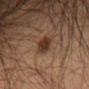Q: Was this lesion biopsied?
A: catalogued during a skin exam; not biopsied
Q: What is the imaging modality?
A: ~15 mm crop, total-body skin-cancer survey
Q: Who is the patient?
A: male, approximately 35 years of age
Q: Where on the body is the lesion?
A: the lower back
Q: What did automated image analysis measure?
A: a lesion area of about 5.5 mm² and an outline eccentricity of about 0.85 (0 = round, 1 = elongated); an average lesion color of about L≈31 a*≈17 b*≈25 (CIELAB), roughly 10 lightness units darker than nearby skin, and a normalized lesion–skin contrast near 9.5; a border-irregularity index near 2.5/10, a within-lesion color-variation index near 2/10, and radial color variation of about 0.5
Q: How large is the lesion?
A: ~4 mm (longest diameter)